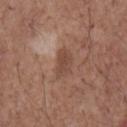{
  "biopsy_status": "not biopsied; imaged during a skin examination",
  "site": "front of the torso",
  "lighting": "white-light",
  "patient": {
    "sex": "male",
    "age_approx": 70
  },
  "automated_metrics": {
    "area_mm2_approx": 5.5,
    "eccentricity": 0.85,
    "shape_asymmetry": 0.25,
    "vs_skin_darker_L": 8.0,
    "vs_skin_contrast_norm": 6.0,
    "nevus_likeness_0_100": 0,
    "lesion_detection_confidence_0_100": 100
  },
  "image": {
    "source": "total-body photography crop",
    "field_of_view_mm": 15
  }
}